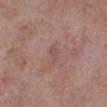<case>
  <biopsy_status>not biopsied; imaged during a skin examination</biopsy_status>
  <site>left lower leg</site>
  <image>
    <source>total-body photography crop</source>
    <field_of_view_mm>15</field_of_view_mm>
  </image>
  <patient>
    <sex>male</sex>
    <age_approx>75</age_approx>
  </patient>
</case>